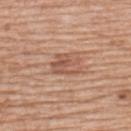Imaged during a routine full-body skin examination; the lesion was not biopsied and no histopathology is available. A female patient, about 50 years old. Located on the upper back. The tile uses white-light illumination. The recorded lesion diameter is about 3.5 mm. Automated tile analysis of the lesion measured a footprint of about 7 mm² and a symmetry-axis asymmetry near 0.4. It also reported a border-irregularity index near 7.5/10, a color-variation rating of about 4.5/10, and radial color variation of about 1.5. It also reported a classifier nevus-likeness of about 0/100 and lesion-presence confidence of about 100/100. A 15 mm crop from a total-body photograph taken for skin-cancer surveillance.